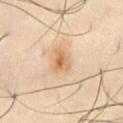biopsy status — total-body-photography surveillance lesion; no biopsy
automated metrics — a classifier nevus-likeness of about 75/100
size — about 3 mm
subject — male, aged around 50
image — 15 mm crop, total-body photography
illumination — cross-polarized
anatomic site — the abdomen